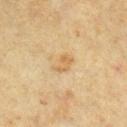Imaged during a routine full-body skin examination; the lesion was not biopsied and no histopathology is available.
Cropped from a total-body skin-imaging series; the visible field is about 15 mm.
Automated tile analysis of the lesion measured border irregularity of about 4.5 on a 0–10 scale, internal color variation of about 1.5 on a 0–10 scale, and radial color variation of about 0.5. It also reported a nevus-likeness score of about 15/100 and lesion-presence confidence of about 100/100.
The patient is a female approximately 55 years of age.
On the right lower leg.
The lesion's longest dimension is about 3 mm.
Imaged with cross-polarized lighting.